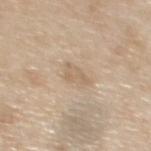- biopsy status · no biopsy performed (imaged during a skin exam)
- lighting · white-light
- patient · female, aged approximately 55
- lesion size · about 3 mm
- image source · total-body-photography crop, ~15 mm field of view
- body site · the upper back